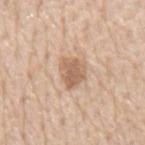Recorded during total-body skin imaging; not selected for excision or biopsy. The lesion-visualizer software estimated a shape eccentricity near 0.55 and a symmetry-axis asymmetry near 0.25. And it measured a mean CIELAB color near L≈62 a*≈18 b*≈31, roughly 11 lightness units darker than nearby skin, and a normalized lesion–skin contrast near 7. The analysis additionally found a classifier nevus-likeness of about 60/100 and a detector confidence of about 100 out of 100 that the crop contains a lesion. A close-up tile cropped from a whole-body skin photograph, about 15 mm across. Captured under white-light illumination. The lesion is located on the mid back. The lesion's longest dimension is about 3.5 mm. A male patient, approximately 75 years of age.